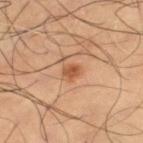No biopsy was performed on this lesion — it was imaged during a full skin examination and was not determined to be concerning. A male subject in their mid-60s. A lesion tile, about 15 mm wide, cut from a 3D total-body photograph. On the left thigh. The tile uses cross-polarized illumination. The lesion's longest dimension is about 2 mm.